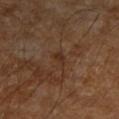The lesion was tiled from a total-body skin photograph and was not biopsied. The lesion is located on the right forearm. A male subject approximately 85 years of age or older. Captured under cross-polarized illumination. Cropped from a whole-body photographic skin survey; the tile spans about 15 mm. The lesion-visualizer software estimated a footprint of about 3 mm², an outline eccentricity of about 0.85 (0 = round, 1 = elongated), and a shape-asymmetry score of about 0.35 (0 = symmetric). And it measured an average lesion color of about L≈29 a*≈17 b*≈26 (CIELAB), about 5 CIELAB-L* units darker than the surrounding skin, and a normalized border contrast of about 5.5. It also reported an automated nevus-likeness rating near 0 out of 100 and a detector confidence of about 100 out of 100 that the crop contains a lesion. The lesion's longest dimension is about 2.5 mm.